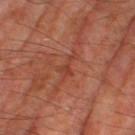Image and clinical context:
About 2.5 mm across. A male patient about 70 years old. From the right thigh. The total-body-photography lesion software estimated a lesion color around L≈41 a*≈28 b*≈31 in CIELAB, about 6 CIELAB-L* units darker than the surrounding skin, and a normalized lesion–skin contrast near 5. The software also gave a color-variation rating of about 0.5/10 and peripheral color asymmetry of about 0. The tile uses cross-polarized illumination. A close-up tile cropped from a whole-body skin photograph, about 15 mm across.